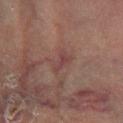The lesion was photographed on a routine skin check and not biopsied; there is no pathology result. A region of skin cropped from a whole-body photographic capture, roughly 15 mm wide. The lesion is located on the leg. Measured at roughly 3 mm in maximum diameter. A male patient aged 53 to 57. The tile uses cross-polarized illumination. The total-body-photography lesion software estimated an eccentricity of roughly 0.9 and a symmetry-axis asymmetry near 0.35.